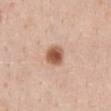This lesion was catalogued during total-body skin photography and was not selected for biopsy. A 15 mm close-up extracted from a 3D total-body photography capture. Located on the abdomen. The subject is a female approximately 45 years of age.